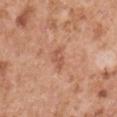site: the arm
patient: male, in their mid-50s
size: ~3 mm (longest diameter)
illumination: white-light
image source: ~15 mm crop, total-body skin-cancer survey